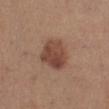Notes:
• biopsy status · imaged on a skin check; not biopsied
• tile lighting · white-light illumination
• size · ~4 mm (longest diameter)
• image · total-body-photography crop, ~15 mm field of view
• site · the right lower leg
• patient · female, in their 40s